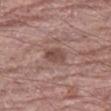Impression: Recorded during total-body skin imaging; not selected for excision or biopsy. Image and clinical context: Captured under white-light illumination. The lesion's longest dimension is about 2.5 mm. From the left thigh. A male subject, approximately 70 years of age. Cropped from a whole-body photographic skin survey; the tile spans about 15 mm. The lesion-visualizer software estimated an average lesion color of about L≈47 a*≈20 b*≈23 (CIELAB), about 10 CIELAB-L* units darker than the surrounding skin, and a lesion-to-skin contrast of about 7.5 (normalized; higher = more distinct). The analysis additionally found a color-variation rating of about 3/10 and a peripheral color-asymmetry measure near 1.